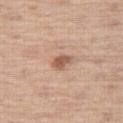  biopsy_status: not biopsied; imaged during a skin examination